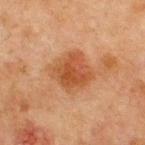Part of a total-body skin-imaging series; this lesion was reviewed on a skin check and was not flagged for biopsy. A male patient in their mid- to late 60s. On the chest. A roughly 15 mm field-of-view crop from a total-body skin photograph.